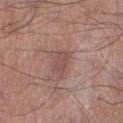Context: A male subject, aged around 70. About 3 mm across. Cropped from a whole-body photographic skin survey; the tile spans about 15 mm. An algorithmic analysis of the crop reported a color-variation rating of about 1.5/10 and a peripheral color-asymmetry measure near 0.5. And it measured lesion-presence confidence of about 100/100. From the right lower leg.